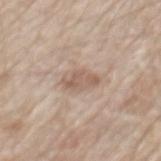This lesion was catalogued during total-body skin photography and was not selected for biopsy. The total-body-photography lesion software estimated border irregularity of about 2.5 on a 0–10 scale, a color-variation rating of about 3/10, and radial color variation of about 1. This image is a 15 mm lesion crop taken from a total-body photograph. A male subject approximately 80 years of age. Imaged with white-light lighting. The lesion is on the mid back.